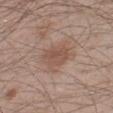Q: Is there a histopathology result?
A: catalogued during a skin exam; not biopsied
Q: What lighting was used for the tile?
A: white-light illumination
Q: What is the lesion's diameter?
A: ~3.5 mm (longest diameter)
Q: Automated lesion metrics?
A: a lesion area of about 6.5 mm², an eccentricity of roughly 0.75, and a symmetry-axis asymmetry near 0.2; a lesion color around L≈51 a*≈18 b*≈26 in CIELAB, a lesion–skin lightness drop of about 8, and a normalized lesion–skin contrast near 6
Q: What kind of image is this?
A: 15 mm crop, total-body photography
Q: Patient demographics?
A: male, about 35 years old
Q: What is the anatomic site?
A: the left lower leg